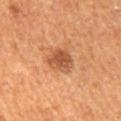The lesion was photographed on a routine skin check and not biopsied; there is no pathology result. A male patient, in their mid- to late 60s. Cropped from a total-body skin-imaging series; the visible field is about 15 mm. Captured under cross-polarized illumination. Longest diameter approximately 3.5 mm. Automated image analysis of the tile measured a lesion area of about 8 mm². And it measured an average lesion color of about L≈51 a*≈26 b*≈36 (CIELAB) and a normalized lesion–skin contrast near 7.5. It also reported a within-lesion color-variation index near 3/10 and a peripheral color-asymmetry measure near 1. The analysis additionally found a nevus-likeness score of about 65/100 and a lesion-detection confidence of about 100/100. The lesion is on the mid back.